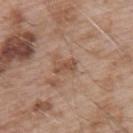• workup — total-body-photography surveillance lesion; no biopsy
• patient — male, roughly 55 years of age
• size — ≈3 mm
• lighting — white-light
• TBP lesion metrics — about 9 CIELAB-L* units darker than the surrounding skin
• body site — the upper back
• acquisition — ~15 mm tile from a whole-body skin photo